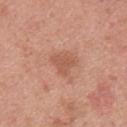Impression: No biopsy was performed on this lesion — it was imaged during a full skin examination and was not determined to be concerning. Background: The lesion is located on the upper back. A 15 mm close-up tile from a total-body photography series done for melanoma screening. A male subject, aged around 25.